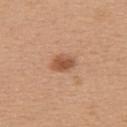| feature | finding |
|---|---|
| follow-up | catalogued during a skin exam; not biopsied |